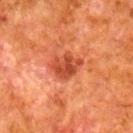workup: no biopsy performed (imaged during a skin exam)
subject: male, approximately 80 years of age
site: the leg
image: 15 mm crop, total-body photography
image-analysis metrics: an area of roughly 8 mm², an outline eccentricity of about 0.7 (0 = round, 1 = elongated), and a symmetry-axis asymmetry near 0.25; a lesion color around L≈39 a*≈30 b*≈33 in CIELAB and roughly 9 lightness units darker than nearby skin; border irregularity of about 2.5 on a 0–10 scale, internal color variation of about 3.5 on a 0–10 scale, and radial color variation of about 1.5
lighting: cross-polarized
diameter: ~4 mm (longest diameter)